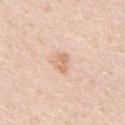Case summary:
- follow-up: imaged on a skin check; not biopsied
- diameter: ~2.5 mm (longest diameter)
- TBP lesion metrics: an area of roughly 3.5 mm², an eccentricity of roughly 0.8, and a shape-asymmetry score of about 0.2 (0 = symmetric); a within-lesion color-variation index near 3/10 and radial color variation of about 1; a classifier nevus-likeness of about 5/100 and a lesion-detection confidence of about 100/100
- subject: male, about 50 years old
- imaging modality: ~15 mm tile from a whole-body skin photo
- anatomic site: the back
- illumination: white-light illumination